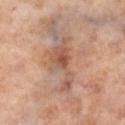follow-up: total-body-photography surveillance lesion; no biopsy.